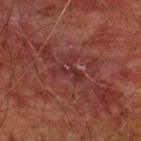Impression:
The lesion was photographed on a routine skin check and not biopsied; there is no pathology result.
Image and clinical context:
A male subject, in their 60s. A lesion tile, about 15 mm wide, cut from a 3D total-body photograph. The lesion is on the upper back. The lesion's longest dimension is about 4 mm. Imaged with cross-polarized lighting.